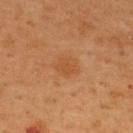biopsy_status: not biopsied; imaged during a skin examination
site: upper back
patient:
  sex: male
  age_approx: 55
image:
  source: total-body photography crop
  field_of_view_mm: 15
automated_metrics:
  vs_skin_darker_L: 5.0
  vs_skin_contrast_norm: 5.0